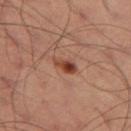Q: Was this lesion biopsied?
A: imaged on a skin check; not biopsied
Q: What are the patient's age and sex?
A: male, roughly 35 years of age
Q: Where on the body is the lesion?
A: the leg
Q: How was this image acquired?
A: 15 mm crop, total-body photography
Q: What lighting was used for the tile?
A: cross-polarized illumination
Q: Automated lesion metrics?
A: a lesion area of about 4 mm², a shape eccentricity near 0.85, and a shape-asymmetry score of about 0.25 (0 = symmetric); a mean CIELAB color near L≈41 a*≈24 b*≈30, roughly 12 lightness units darker than nearby skin, and a normalized border contrast of about 9.5; a border-irregularity rating of about 2.5/10, a color-variation rating of about 6/10, and a peripheral color-asymmetry measure near 2
Q: What is the lesion's diameter?
A: ~2.5 mm (longest diameter)